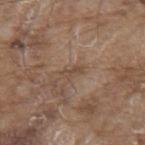Assessment: The lesion was photographed on a routine skin check and not biopsied; there is no pathology result. Clinical summary: A male subject, aged around 80. A 15 mm close-up tile from a total-body photography series done for melanoma screening. An algorithmic analysis of the crop reported a lesion area of about 2.5 mm² and a shape eccentricity near 0.9. The analysis additionally found a lesion–skin lightness drop of about 7. The analysis additionally found a nevus-likeness score of about 0/100 and lesion-presence confidence of about 60/100. The tile uses white-light illumination. The lesion is located on the mid back. The lesion's longest dimension is about 3 mm.